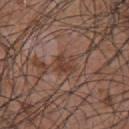notes: total-body-photography surveillance lesion; no biopsy | automated lesion analysis: a lesion area of about 5.5 mm², an eccentricity of roughly 0.45, and a symmetry-axis asymmetry near 0.2; a border-irregularity rating of about 2.5/10, a within-lesion color-variation index near 2.5/10, and radial color variation of about 1; lesion-presence confidence of about 85/100 | body site: the chest | diameter: ≈3 mm | imaging modality: ~15 mm crop, total-body skin-cancer survey | patient: male, in their mid- to late 50s | lighting: white-light illumination.